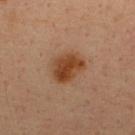* follow-up: imaged on a skin check; not biopsied
* lighting: cross-polarized
* lesion diameter: about 4 mm
* body site: the upper back
* image-analysis metrics: a mean CIELAB color near L≈39 a*≈20 b*≈32, about 10 CIELAB-L* units darker than the surrounding skin, and a normalized lesion–skin contrast near 9.5; a border-irregularity rating of about 2/10, internal color variation of about 4.5 on a 0–10 scale, and radial color variation of about 1.5
* image source: total-body-photography crop, ~15 mm field of view
* patient: male, aged approximately 35